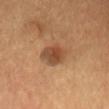follow-up: total-body-photography surveillance lesion; no biopsy
diameter: ~4 mm (longest diameter)
image: ~15 mm tile from a whole-body skin photo
illumination: cross-polarized illumination
subject: male, about 55 years old
TBP lesion metrics: a lesion color around L≈48 a*≈21 b*≈34 in CIELAB and a lesion-to-skin contrast of about 8.5 (normalized; higher = more distinct); a border-irregularity rating of about 1.5/10, internal color variation of about 5.5 on a 0–10 scale, and a peripheral color-asymmetry measure near 2
body site: the front of the torso